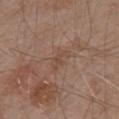A male subject approximately 55 years of age.
From the upper back.
The total-body-photography lesion software estimated a lesion area of about 3 mm², a shape eccentricity near 0.85, and a symmetry-axis asymmetry near 0.45. It also reported a nevus-likeness score of about 0/100 and lesion-presence confidence of about 100/100.
A close-up tile cropped from a whole-body skin photograph, about 15 mm across.
The lesion's longest dimension is about 2.5 mm.